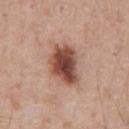Assessment: No biopsy was performed on this lesion — it was imaged during a full skin examination and was not determined to be concerning. Context: A lesion tile, about 15 mm wide, cut from a 3D total-body photograph. Imaged with white-light lighting. A male patient, aged around 55. From the chest. Longest diameter approximately 5 mm.